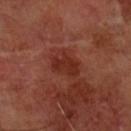follow-up: imaged on a skin check; not biopsied